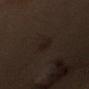The lesion was photographed on a routine skin check and not biopsied; there is no pathology result. Imaged with cross-polarized lighting. The lesion is located on the left upper arm. A region of skin cropped from a whole-body photographic capture, roughly 15 mm wide. A male patient, about 50 years old. About 2.5 mm across.